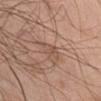notes: total-body-photography surveillance lesion; no biopsy.